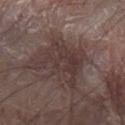Part of a total-body skin-imaging series; this lesion was reviewed on a skin check and was not flagged for biopsy. Automated image analysis of the tile measured a footprint of about 21 mm², a shape eccentricity near 0.7, and two-axis asymmetry of about 0.45. It also reported an average lesion color of about L≈35 a*≈15 b*≈17 (CIELAB) and a normalized border contrast of about 7. The software also gave a border-irregularity rating of about 6.5/10 and radial color variation of about 1.5. This image is a 15 mm lesion crop taken from a total-body photograph. The tile uses white-light illumination. The lesion is on the right arm. About 6.5 mm across. The subject is a male roughly 80 years of age.